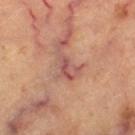follow-up — total-body-photography surveillance lesion; no biopsy
acquisition — total-body-photography crop, ~15 mm field of view
diameter — ~3.5 mm (longest diameter)
illumination — cross-polarized
TBP lesion metrics — an eccentricity of roughly 0.8 and two-axis asymmetry of about 0.4; a mean CIELAB color near L≈53 a*≈25 b*≈25, a lesion–skin lightness drop of about 9, and a normalized border contrast of about 7
body site — the leg
patient — roughly 60 years of age